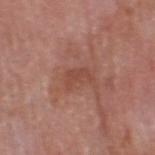Assessment: Captured during whole-body skin photography for melanoma surveillance; the lesion was not biopsied. Context: The lesion is located on the head or neck. This image is a 15 mm lesion crop taken from a total-body photograph. The lesion-visualizer software estimated a lesion area of about 4 mm², a shape eccentricity near 0.8, and a symmetry-axis asymmetry near 0.45. The analysis additionally found a mean CIELAB color near L≈47 a*≈24 b*≈28, a lesion–skin lightness drop of about 6, and a normalized lesion–skin contrast near 5. And it measured an automated nevus-likeness rating near 0 out of 100. A male patient, aged around 60. Longest diameter approximately 3 mm.